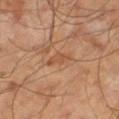Assessment: Imaged during a routine full-body skin examination; the lesion was not biopsied and no histopathology is available. Acquisition and patient details: A male subject aged 63–67. A close-up tile cropped from a whole-body skin photograph, about 15 mm across.